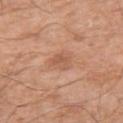No biopsy was performed on this lesion — it was imaged during a full skin examination and was not determined to be concerning. Located on the left upper arm. Automated tile analysis of the lesion measured an average lesion color of about L≈57 a*≈23 b*≈32 (CIELAB), about 8 CIELAB-L* units darker than the surrounding skin, and a normalized lesion–skin contrast near 5.5. The analysis additionally found a nevus-likeness score of about 0/100 and a detector confidence of about 100 out of 100 that the crop contains a lesion. The tile uses white-light illumination. The patient is a male in their mid- to late 60s. Cropped from a whole-body photographic skin survey; the tile spans about 15 mm. About 2.5 mm across.